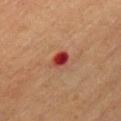The lesion was tiled from a total-body skin photograph and was not biopsied. Measured at roughly 2.5 mm in maximum diameter. A 15 mm close-up tile from a total-body photography series done for melanoma screening. A female subject, in their mid-50s. On the chest. The tile uses cross-polarized illumination.